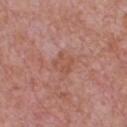<case>
<biopsy_status>not biopsied; imaged during a skin examination</biopsy_status>
<site>chest</site>
<patient>
  <sex>male</sex>
  <age_approx>60</age_approx>
</patient>
<lighting>white-light</lighting>
<lesion_size>
  <long_diameter_mm_approx>2.5</long_diameter_mm_approx>
</lesion_size>
<image>
  <source>total-body photography crop</source>
  <field_of_view_mm>15</field_of_view_mm>
</image>
</case>